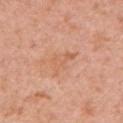Part of a total-body skin-imaging series; this lesion was reviewed on a skin check and was not flagged for biopsy.
Imaged with white-light lighting.
A female patient approximately 40 years of age.
Automated tile analysis of the lesion measured an average lesion color of about L≈62 a*≈24 b*≈35 (CIELAB), about 6 CIELAB-L* units darker than the surrounding skin, and a normalized lesion–skin contrast near 4.5. The software also gave lesion-presence confidence of about 100/100.
Measured at roughly 3.5 mm in maximum diameter.
A roughly 15 mm field-of-view crop from a total-body skin photograph.
From the arm.